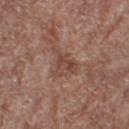Q: Was a biopsy performed?
A: catalogued during a skin exam; not biopsied
Q: Lesion location?
A: the right thigh
Q: What kind of image is this?
A: ~15 mm tile from a whole-body skin photo
Q: How large is the lesion?
A: ≈3.5 mm
Q: Illumination type?
A: white-light
Q: Who is the patient?
A: female, in their 80s